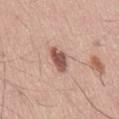Clinical impression:
The lesion was photographed on a routine skin check and not biopsied; there is no pathology result.
Clinical summary:
A close-up tile cropped from a whole-body skin photograph, about 15 mm across. The lesion is located on the back. A male subject, aged approximately 60. Measured at roughly 3.5 mm in maximum diameter.